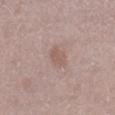biopsy status = imaged on a skin check; not biopsied
lighting = white-light
image source = ~15 mm tile from a whole-body skin photo
subject = female, aged approximately 50
size = ~3 mm (longest diameter)
location = the right lower leg